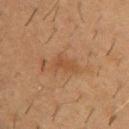illumination: cross-polarized | subject: male, roughly 55 years of age | automated metrics: a lesion color around L≈42 a*≈19 b*≈32 in CIELAB, roughly 6 lightness units darker than nearby skin, and a normalized lesion–skin contrast near 5.5 | image: 15 mm crop, total-body photography | lesion size: ≈3 mm | body site: the chest.